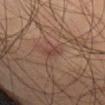From the abdomen. The lesion-visualizer software estimated a classifier nevus-likeness of about 0/100. Approximately 2.5 mm at its widest. Captured under cross-polarized illumination. A male patient aged around 60. A 15 mm crop from a total-body photograph taken for skin-cancer surveillance.